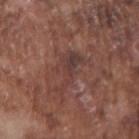Background: The lesion is on the right upper arm. Automated tile analysis of the lesion measured a footprint of about 8 mm² and two-axis asymmetry of about 0.6. And it measured an average lesion color of about L≈38 a*≈20 b*≈21 (CIELAB) and a normalized lesion–skin contrast near 6.5. And it measured a classifier nevus-likeness of about 0/100 and a detector confidence of about 60 out of 100 that the crop contains a lesion. This is a white-light tile. Cropped from a total-body skin-imaging series; the visible field is about 15 mm. A male subject approximately 75 years of age.